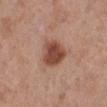Part of a total-body skin-imaging series; this lesion was reviewed on a skin check and was not flagged for biopsy.
Measured at roughly 3.5 mm in maximum diameter.
A 15 mm crop from a total-body photograph taken for skin-cancer surveillance.
Automated image analysis of the tile measured an average lesion color of about L≈47 a*≈23 b*≈28 (CIELAB) and a lesion–skin lightness drop of about 14. And it measured an automated nevus-likeness rating near 95 out of 100 and lesion-presence confidence of about 100/100.
On the left lower leg.
A female subject aged approximately 40.